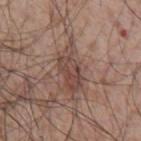Imaged during a routine full-body skin examination; the lesion was not biopsied and no histopathology is available.
A male subject, aged around 55.
The lesion is located on the chest.
Cropped from a whole-body photographic skin survey; the tile spans about 15 mm.